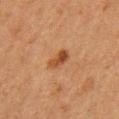| field | value |
|---|---|
| follow-up | no biopsy performed (imaged during a skin exam) |
| lesion size | ~3 mm (longest diameter) |
| subject | male, in their mid- to late 60s |
| anatomic site | the chest |
| TBP lesion metrics | an outline eccentricity of about 0.85 (0 = round, 1 = elongated) and two-axis asymmetry of about 0.25; about 10 CIELAB-L* units darker than the surrounding skin; border irregularity of about 2.5 on a 0–10 scale, internal color variation of about 4.5 on a 0–10 scale, and radial color variation of about 1.5; a nevus-likeness score of about 80/100 and a lesion-detection confidence of about 100/100 |
| acquisition | 15 mm crop, total-body photography |